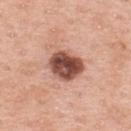Findings:
* workup · total-body-photography surveillance lesion; no biopsy
* subject · male, in their 60s
* imaging modality · ~15 mm tile from a whole-body skin photo
* body site · the upper back
* image-analysis metrics · a lesion area of about 12 mm² and a symmetry-axis asymmetry near 0.2; a lesion–skin lightness drop of about 20 and a lesion-to-skin contrast of about 13 (normalized; higher = more distinct); a nevus-likeness score of about 35/100
* lesion size · about 4.5 mm